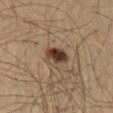Longest diameter approximately 4 mm. A male patient about 65 years old. This image is a 15 mm lesion crop taken from a total-body photograph. The tile uses cross-polarized illumination. The lesion is on the left upper arm.